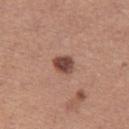Assessment:
No biopsy was performed on this lesion — it was imaged during a full skin examination and was not determined to be concerning.
Image and clinical context:
The lesion's longest dimension is about 3 mm. This is a white-light tile. The patient is a female in their mid-30s. The lesion is on the leg. A 15 mm crop from a total-body photograph taken for skin-cancer surveillance.